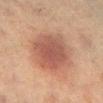{
  "biopsy_status": "not biopsied; imaged during a skin examination",
  "site": "left lower leg",
  "image": {
    "source": "total-body photography crop",
    "field_of_view_mm": 15
  },
  "patient": {
    "sex": "female",
    "age_approx": 55
  }
}